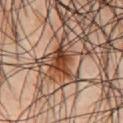Recorded during total-body skin imaging; not selected for excision or biopsy. The lesion is located on the chest. A lesion tile, about 15 mm wide, cut from a 3D total-body photograph. Approximately 4 mm at its widest. The patient is a male roughly 45 years of age. The tile uses cross-polarized illumination. The lesion-visualizer software estimated a lesion color around L≈39 a*≈22 b*≈31 in CIELAB, roughly 15 lightness units darker than nearby skin, and a lesion-to-skin contrast of about 12 (normalized; higher = more distinct). The software also gave a border-irregularity rating of about 4/10 and a color-variation rating of about 6/10. It also reported a nevus-likeness score of about 90/100 and lesion-presence confidence of about 90/100.